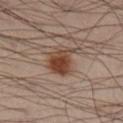Recorded during total-body skin imaging; not selected for excision or biopsy. A 15 mm crop from a total-body photograph taken for skin-cancer surveillance. The lesion is on the right lower leg. A male patient, aged approximately 40. Measured at roughly 3.5 mm in maximum diameter. This is a cross-polarized tile.